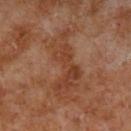notes — total-body-photography surveillance lesion; no biopsy | illumination — cross-polarized | subject — male, in their 70s | body site — the left lower leg | TBP lesion metrics — a footprint of about 17 mm² and an outline eccentricity of about 0.95 (0 = round, 1 = elongated); roughly 7 lightness units darker than nearby skin and a normalized lesion–skin contrast near 6; border irregularity of about 8 on a 0–10 scale and radial color variation of about 1 | imaging modality — total-body-photography crop, ~15 mm field of view | lesion size — about 8 mm.